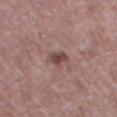Automated image analysis of the tile measured a footprint of about 4 mm² and an eccentricity of roughly 0.75. It also reported a mean CIELAB color near L≈45 a*≈20 b*≈20. It also reported border irregularity of about 2 on a 0–10 scale and radial color variation of about 1.5.
A male patient, approximately 70 years of age.
The lesion is located on the left thigh.
The tile uses white-light illumination.
Cropped from a total-body skin-imaging series; the visible field is about 15 mm.
Longest diameter approximately 2.5 mm.